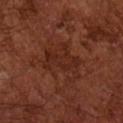Clinical impression: Part of a total-body skin-imaging series; this lesion was reviewed on a skin check and was not flagged for biopsy. Context: A roughly 15 mm field-of-view crop from a total-body skin photograph. A male subject, approximately 65 years of age. The lesion is on the left arm. The lesion's longest dimension is about 4.5 mm. The tile uses cross-polarized illumination. An algorithmic analysis of the crop reported a lesion area of about 8 mm² and a symmetry-axis asymmetry near 0.3. And it measured an average lesion color of about L≈25 a*≈23 b*≈26 (CIELAB), about 5 CIELAB-L* units darker than the surrounding skin, and a normalized border contrast of about 5.5. It also reported a border-irregularity index near 4.5/10 and a peripheral color-asymmetry measure near 1. It also reported a classifier nevus-likeness of about 5/100.